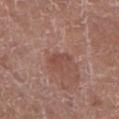<case>
<biopsy_status>not biopsied; imaged during a skin examination</biopsy_status>
<lesion_size>
  <long_diameter_mm_approx>2.5</long_diameter_mm_approx>
</lesion_size>
<image>
  <source>total-body photography crop</source>
  <field_of_view_mm>15</field_of_view_mm>
</image>
<patient>
  <sex>female</sex>
  <age_approx>80</age_approx>
</patient>
<automated_metrics>
  <peripheral_color_asymmetry>0.0</peripheral_color_asymmetry>
</automated_metrics>
<lighting>white-light</lighting>
<site>right lower leg</site>
</case>